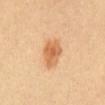Assessment: Recorded during total-body skin imaging; not selected for excision or biopsy. Context: Captured under cross-polarized illumination. The total-body-photography lesion software estimated a lesion area of about 8.5 mm², an eccentricity of roughly 0.7, and two-axis asymmetry of about 0.2. The software also gave a border-irregularity index near 2/10, internal color variation of about 4 on a 0–10 scale, and peripheral color asymmetry of about 1. From the abdomen. The subject is a female aged 33 to 37. A close-up tile cropped from a whole-body skin photograph, about 15 mm across.